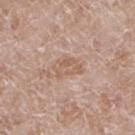Impression: Imaged during a routine full-body skin examination; the lesion was not biopsied and no histopathology is available. Clinical summary: The tile uses white-light illumination. The recorded lesion diameter is about 4 mm. A 15 mm close-up tile from a total-body photography series done for melanoma screening. A male subject, aged 58 to 62. The lesion is on the left lower leg.